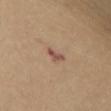Q: Is there a histopathology result?
A: no biopsy performed (imaged during a skin exam)
Q: How was this image acquired?
A: total-body-photography crop, ~15 mm field of view
Q: What lighting was used for the tile?
A: cross-polarized
Q: Lesion size?
A: ≈2.5 mm
Q: Where on the body is the lesion?
A: the abdomen
Q: What are the patient's age and sex?
A: female, aged approximately 55